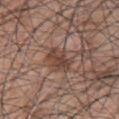<record>
<biopsy_status>not biopsied; imaged during a skin examination</biopsy_status>
</record>